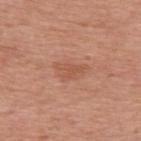workup: imaged on a skin check; not biopsied
body site: the upper back
lighting: white-light illumination
image: ~15 mm tile from a whole-body skin photo
patient: female, aged approximately 40
automated metrics: a lesion area of about 6 mm², a shape eccentricity near 0.85, and a shape-asymmetry score of about 0.4 (0 = symmetric); a mean CIELAB color near L≈55 a*≈25 b*≈32, about 7 CIELAB-L* units darker than the surrounding skin, and a lesion-to-skin contrast of about 5 (normalized; higher = more distinct); border irregularity of about 4 on a 0–10 scale, internal color variation of about 2 on a 0–10 scale, and radial color variation of about 0.5; a classifier nevus-likeness of about 0/100